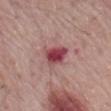biopsy status — imaged on a skin check; not biopsied
lesion size — ~3.5 mm (longest diameter)
subject — male, aged 68 to 72
acquisition — ~15 mm crop, total-body skin-cancer survey
site — the mid back